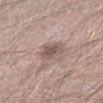Imaged during a routine full-body skin examination; the lesion was not biopsied and no histopathology is available. A male patient aged 28–32. A region of skin cropped from a whole-body photographic capture, roughly 15 mm wide.